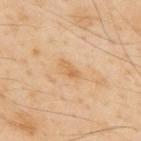| key | value |
|---|---|
| follow-up | imaged on a skin check; not biopsied |
| subject | male, approximately 55 years of age |
| automated metrics | a lesion color around L≈65 a*≈20 b*≈41 in CIELAB, a lesion–skin lightness drop of about 8, and a normalized border contrast of about 6; a within-lesion color-variation index near 0/10 |
| body site | the upper back |
| lesion size | ~3 mm (longest diameter) |
| image | ~15 mm tile from a whole-body skin photo |
| tile lighting | cross-polarized illumination |